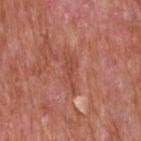site: head or neck
patient:
  sex: male
  age_approx: 65
lesion_size:
  long_diameter_mm_approx: 4.5
lighting: white-light
image:
  source: total-body photography crop
  field_of_view_mm: 15